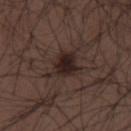The lesion was photographed on a routine skin check and not biopsied; there is no pathology result.